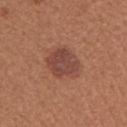<tbp_lesion>
  <biopsy_status>not biopsied; imaged during a skin examination</biopsy_status>
  <patient>
    <sex>female</sex>
    <age_approx>40</age_approx>
  </patient>
  <lesion_size>
    <long_diameter_mm_approx>4.0</long_diameter_mm_approx>
  </lesion_size>
  <image>
    <source>total-body photography crop</source>
    <field_of_view_mm>15</field_of_view_mm>
  </image>
  <site>right upper arm</site>
  <lighting>white-light</lighting>
</tbp_lesion>